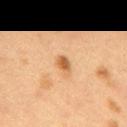subject = female, approximately 40 years of age | anatomic site = the back | imaging modality = ~15 mm crop, total-body skin-cancer survey.